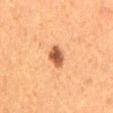* workup · imaged on a skin check; not biopsied